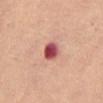Impression:
The lesion was tiled from a total-body skin photograph and was not biopsied.
Context:
From the abdomen. A female subject, roughly 65 years of age. Longest diameter approximately 2.5 mm. A 15 mm close-up extracted from a 3D total-body photography capture.